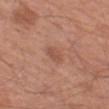  biopsy_status: not biopsied; imaged during a skin examination
  automated_metrics:
    vs_skin_darker_L: 7.0
    vs_skin_contrast_norm: 5.5
  site: left thigh
  patient:
    sex: male
    age_approx: 65
  lesion_size:
    long_diameter_mm_approx: 2.5
  image:
    source: total-body photography crop
    field_of_view_mm: 15